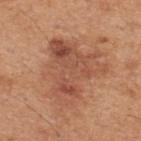Impression:
The lesion was tiled from a total-body skin photograph and was not biopsied.
Background:
The lesion is on the upper back. A 15 mm close-up tile from a total-body photography series done for melanoma screening. Measured at roughly 7.5 mm in maximum diameter. The lesion-visualizer software estimated an area of roughly 31 mm² and an outline eccentricity of about 0.55 (0 = round, 1 = elongated). The software also gave a border-irregularity rating of about 6.5/10, a color-variation rating of about 6.5/10, and peripheral color asymmetry of about 2.5. And it measured an automated nevus-likeness rating near 20 out of 100. A male subject approximately 45 years of age. This is a white-light tile.